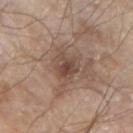biopsy status: catalogued during a skin exam; not biopsied
body site: the arm
lesion size: ≈4 mm
illumination: white-light illumination
image: total-body-photography crop, ~15 mm field of view
patient: male, aged 78–82